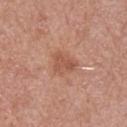Assessment:
Captured during whole-body skin photography for melanoma surveillance; the lesion was not biopsied.
Context:
An algorithmic analysis of the crop reported a classifier nevus-likeness of about 0/100 and lesion-presence confidence of about 100/100. The recorded lesion diameter is about 3 mm. A close-up tile cropped from a whole-body skin photograph, about 15 mm across. Captured under white-light illumination. The patient is a male approximately 70 years of age. The lesion is located on the arm.